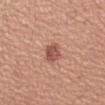Impression:
Imaged during a routine full-body skin examination; the lesion was not biopsied and no histopathology is available.
Background:
The lesion is located on the left forearm. A 15 mm close-up tile from a total-body photography series done for melanoma screening. A female subject aged 43 to 47.